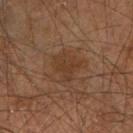This lesion was catalogued during total-body skin photography and was not selected for biopsy. The tile uses cross-polarized illumination. The patient is a male aged around 65. Approximately 3.5 mm at its widest. A region of skin cropped from a whole-body photographic capture, roughly 15 mm wide. On the right upper arm.